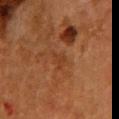Clinical impression:
Imaged during a routine full-body skin examination; the lesion was not biopsied and no histopathology is available.
Acquisition and patient details:
A roughly 15 mm field-of-view crop from a total-body skin photograph. The lesion-visualizer software estimated a lesion area of about 3.5 mm², an eccentricity of roughly 0.7, and two-axis asymmetry of about 0.65. It also reported roughly 4 lightness units darker than nearby skin and a normalized border contrast of about 4.5. A female subject, approximately 50 years of age. Measured at roughly 3 mm in maximum diameter. The lesion is on the front of the torso.